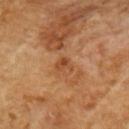Notes:
- follow-up: total-body-photography surveillance lesion; no biopsy